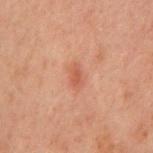Measured at roughly 2.5 mm in maximum diameter.
A 15 mm close-up extracted from a 3D total-body photography capture.
The total-body-photography lesion software estimated about 6 CIELAB-L* units darker than the surrounding skin. The software also gave a nevus-likeness score of about 10/100 and a detector confidence of about 100 out of 100 that the crop contains a lesion.
The lesion is located on the chest.
The subject is a male aged 58 to 62.